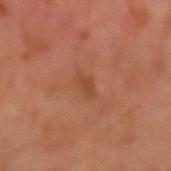The lesion was photographed on a routine skin check and not biopsied; there is no pathology result. Approximately 3 mm at its widest. An algorithmic analysis of the crop reported an eccentricity of roughly 0.85 and two-axis asymmetry of about 0.2. It also reported an automated nevus-likeness rating near 0 out of 100 and a detector confidence of about 100 out of 100 that the crop contains a lesion. A 15 mm close-up tile from a total-body photography series done for melanoma screening. A male subject roughly 65 years of age. The tile uses cross-polarized illumination.